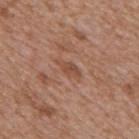Findings:
- diameter: ≈3 mm
- subject: male, approximately 65 years of age
- illumination: white-light
- TBP lesion metrics: a shape eccentricity near 0.9 and a shape-asymmetry score of about 0.3 (0 = symmetric); an average lesion color of about L≈48 a*≈22 b*≈30 (CIELAB), roughly 8 lightness units darker than nearby skin, and a lesion-to-skin contrast of about 6 (normalized; higher = more distinct); a nevus-likeness score of about 0/100 and a lesion-detection confidence of about 85/100
- body site: the back
- imaging modality: total-body-photography crop, ~15 mm field of view